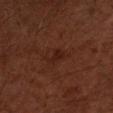Notes:
- patient · male, aged around 60
- image · ~15 mm crop, total-body skin-cancer survey
- tile lighting · cross-polarized
- body site · the left forearm
- size · ~2.5 mm (longest diameter)